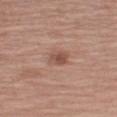Image and clinical context:
Cropped from a whole-body photographic skin survey; the tile spans about 15 mm. The lesion is located on the left upper arm. A male subject aged 58–62.